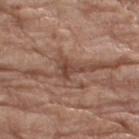Q: Illumination type?
A: white-light
Q: What is the imaging modality?
A: total-body-photography crop, ~15 mm field of view
Q: Automated lesion metrics?
A: a footprint of about 3 mm², an eccentricity of roughly 0.75, and a shape-asymmetry score of about 0.6 (0 = symmetric); a normalized lesion–skin contrast near 8; a border-irregularity rating of about 6/10, a color-variation rating of about 1.5/10, and radial color variation of about 0.5
Q: What is the anatomic site?
A: the right thigh
Q: Who is the patient?
A: female, roughly 75 years of age